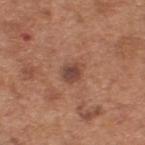Part of a total-body skin-imaging series; this lesion was reviewed on a skin check and was not flagged for biopsy.
Located on the back.
A male subject roughly 65 years of age.
Captured under white-light illumination.
Measured at roughly 2.5 mm in maximum diameter.
A lesion tile, about 15 mm wide, cut from a 3D total-body photograph.